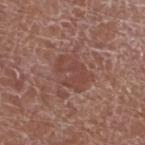A male patient approximately 75 years of age. On the left lower leg. This image is a 15 mm lesion crop taken from a total-body photograph.